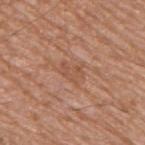Impression:
Imaged during a routine full-body skin examination; the lesion was not biopsied and no histopathology is available.
Acquisition and patient details:
A male patient, aged 63–67. The tile uses white-light illumination. This image is a 15 mm lesion crop taken from a total-body photograph. On the upper back.